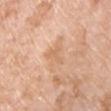Notes:
– follow-up — no biopsy performed (imaged during a skin exam)
– body site — the chest
– imaging modality — 15 mm crop, total-body photography
– subject — male, in their 60s
– TBP lesion metrics — an outline eccentricity of about 0.8 (0 = round, 1 = elongated) and a shape-asymmetry score of about 0.7 (0 = symmetric); a mean CIELAB color near L≈66 a*≈21 b*≈36, about 6 CIELAB-L* units darker than the surrounding skin, and a normalized border contrast of about 4.5
– diameter — ~2.5 mm (longest diameter)